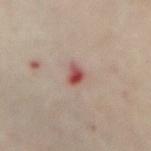Clinical impression:
Imaged during a routine full-body skin examination; the lesion was not biopsied and no histopathology is available.
Clinical summary:
Imaged with cross-polarized lighting. A female patient, aged around 60. The lesion's longest dimension is about 2.5 mm. On the abdomen. Cropped from a whole-body photographic skin survey; the tile spans about 15 mm.